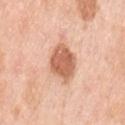Case summary:
– notes: catalogued during a skin exam; not biopsied
– anatomic site: the left upper arm
– lighting: white-light illumination
– image source: ~15 mm tile from a whole-body skin photo
– automated lesion analysis: an automated nevus-likeness rating near 95 out of 100 and a lesion-detection confidence of about 100/100
– subject: female, about 50 years old